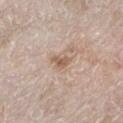Imaged during a routine full-body skin examination; the lesion was not biopsied and no histopathology is available.
A female patient roughly 75 years of age.
The total-body-photography lesion software estimated a shape eccentricity near 0.8. It also reported a normalized border contrast of about 7.5. The software also gave a color-variation rating of about 1/10 and a peripheral color-asymmetry measure near 0.5. It also reported an automated nevus-likeness rating near 0 out of 100.
On the left lower leg.
Measured at roughly 2.5 mm in maximum diameter.
This is a white-light tile.
Cropped from a total-body skin-imaging series; the visible field is about 15 mm.